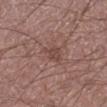acquisition = ~15 mm crop, total-body skin-cancer survey; patient = male, aged 58–62; anatomic site = the left lower leg.